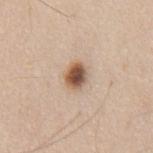This lesion was catalogued during total-body skin photography and was not selected for biopsy.
A 15 mm close-up tile from a total-body photography series done for melanoma screening.
The subject is a male about 60 years old.
Automated tile analysis of the lesion measured a mean CIELAB color near L≈54 a*≈18 b*≈30, roughly 18 lightness units darker than nearby skin, and a normalized lesion–skin contrast near 11.5. It also reported internal color variation of about 7 on a 0–10 scale and a peripheral color-asymmetry measure near 2. The analysis additionally found an automated nevus-likeness rating near 100 out of 100 and lesion-presence confidence of about 100/100.
Located on the mid back.
The tile uses white-light illumination.
About 3 mm across.